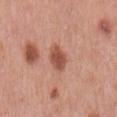| key | value |
|---|---|
| notes | total-body-photography surveillance lesion; no biopsy |
| patient | male, aged 68 to 72 |
| tile lighting | white-light |
| body site | the mid back |
| lesion size | about 3 mm |
| image source | 15 mm crop, total-body photography |